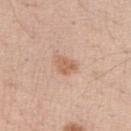Impression: The lesion was photographed on a routine skin check and not biopsied; there is no pathology result. Context: Imaged with white-light lighting. Approximately 3 mm at its widest. Located on the right upper arm. Automated image analysis of the tile measured a footprint of about 4.5 mm², an eccentricity of roughly 0.7, and a shape-asymmetry score of about 0.3 (0 = symmetric). And it measured a mean CIELAB color near L≈62 a*≈20 b*≈32 and a normalized border contrast of about 6.5. It also reported a nevus-likeness score of about 25/100 and a detector confidence of about 100 out of 100 that the crop contains a lesion. Cropped from a total-body skin-imaging series; the visible field is about 15 mm. A female subject, aged 38 to 42.